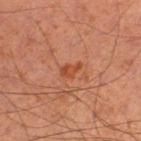Clinical summary:
Imaged with cross-polarized lighting. A male subject about 65 years old. Longest diameter approximately 2.5 mm. A region of skin cropped from a whole-body photographic capture, roughly 15 mm wide. Located on the leg. Automated tile analysis of the lesion measured a lesion area of about 3 mm², a shape eccentricity near 0.8, and a symmetry-axis asymmetry near 0.35. It also reported a border-irregularity rating of about 3.5/10, internal color variation of about 1.5 on a 0–10 scale, and peripheral color asymmetry of about 0.5. And it measured a nevus-likeness score of about 10/100 and lesion-presence confidence of about 100/100.